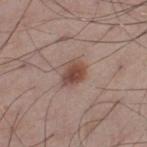Findings:
• follow-up · catalogued during a skin exam; not biopsied
• size · ~3 mm (longest diameter)
• location · the leg
• acquisition · 15 mm crop, total-body photography
• lighting · white-light
• patient · male, in their 60s